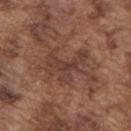Notes:
- biopsy status · catalogued during a skin exam; not biopsied
- illumination · white-light
- image source · total-body-photography crop, ~15 mm field of view
- subject · male, in their mid- to late 70s
- body site · the back